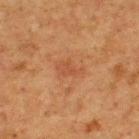Q: What is the imaging modality?
A: ~15 mm tile from a whole-body skin photo
Q: What is the lesion's diameter?
A: about 3 mm
Q: What is the anatomic site?
A: the upper back
Q: How was the tile lit?
A: cross-polarized
Q: What are the patient's age and sex?
A: male, about 75 years old